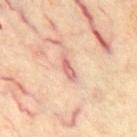{
  "biopsy_status": "not biopsied; imaged during a skin examination",
  "image": {
    "source": "total-body photography crop",
    "field_of_view_mm": 15
  },
  "patient": {
    "sex": "male",
    "age_approx": 60
  },
  "automated_metrics": {
    "nevus_likeness_0_100": 0,
    "lesion_detection_confidence_0_100": 95
  },
  "site": "chest",
  "lesion_size": {
    "long_diameter_mm_approx": 3.0
  }
}